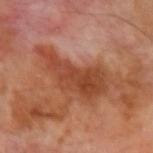Imaged during a routine full-body skin examination; the lesion was not biopsied and no histopathology is available.
The recorded lesion diameter is about 8.5 mm.
From the left upper arm.
This is a cross-polarized tile.
A roughly 15 mm field-of-view crop from a total-body skin photograph.
The patient is a male aged 68–72.